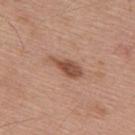image:
  source: total-body photography crop
  field_of_view_mm: 15
automated_metrics:
  area_mm2_approx: 6.0
  eccentricity: 0.9
  shape_asymmetry: 0.35
patient:
  sex: male
  age_approx: 55
site: back
lesion_size:
  long_diameter_mm_approx: 4.5